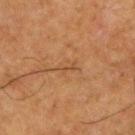notes: catalogued during a skin exam; not biopsied
anatomic site: the right thigh
imaging modality: ~15 mm tile from a whole-body skin photo
subject: male, roughly 80 years of age